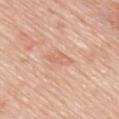Clinical impression:
The lesion was tiled from a total-body skin photograph and was not biopsied.
Context:
Cropped from a whole-body photographic skin survey; the tile spans about 15 mm. The recorded lesion diameter is about 3.5 mm. A male patient in their 80s. Located on the mid back. This is a white-light tile.